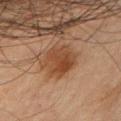<case>
  <biopsy_status>not biopsied; imaged during a skin examination</biopsy_status>
  <lesion_size>
    <long_diameter_mm_approx>4.0</long_diameter_mm_approx>
  </lesion_size>
  <site>left upper arm</site>
  <image>
    <source>total-body photography crop</source>
    <field_of_view_mm>15</field_of_view_mm>
  </image>
  <patient>
    <sex>male</sex>
    <age_approx>65</age_approx>
  </patient>
</case>